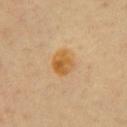– notes · total-body-photography surveillance lesion; no biopsy
– body site · the chest
– image source · total-body-photography crop, ~15 mm field of view
– subject · roughly 60 years of age
– tile lighting · cross-polarized
– lesion size · about 3 mm
– automated lesion analysis · a lesion area of about 7.5 mm² and two-axis asymmetry of about 0.15; an automated nevus-likeness rating near 85 out of 100 and a lesion-detection confidence of about 100/100